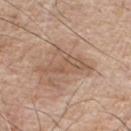Imaged during a routine full-body skin examination; the lesion was not biopsied and no histopathology is available. The lesion-visualizer software estimated a lesion area of about 9.5 mm² and a shape eccentricity near 0.85. It also reported a classifier nevus-likeness of about 0/100 and a detector confidence of about 100 out of 100 that the crop contains a lesion. The recorded lesion diameter is about 6 mm. The tile uses white-light illumination. A close-up tile cropped from a whole-body skin photograph, about 15 mm across. A male subject, approximately 75 years of age. The lesion is located on the chest.